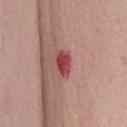Recorded during total-body skin imaging; not selected for excision or biopsy. A female patient, in their 50s. A roughly 15 mm field-of-view crop from a total-body skin photograph. On the back.